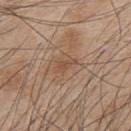Image and clinical context:
The tile uses white-light illumination. The patient is a male in their mid- to late 40s. The lesion is on the upper back. A 15 mm crop from a total-body photograph taken for skin-cancer surveillance.